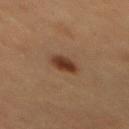Impression:
The lesion was photographed on a routine skin check and not biopsied; there is no pathology result.
Acquisition and patient details:
Cropped from a whole-body photographic skin survey; the tile spans about 15 mm. A male patient, aged approximately 60. From the mid back.